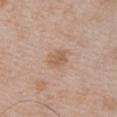| field | value |
|---|---|
| notes | no biopsy performed (imaged during a skin exam) |
| acquisition | 15 mm crop, total-body photography |
| subject | male, aged around 50 |
| tile lighting | white-light |
| site | the chest |
| lesion diameter | ~2.5 mm (longest diameter) |
| TBP lesion metrics | an average lesion color of about L≈58 a*≈18 b*≈31 (CIELAB), roughly 8 lightness units darker than nearby skin, and a lesion-to-skin contrast of about 6 (normalized; higher = more distinct); a border-irregularity index near 2/10 and a peripheral color-asymmetry measure near 1; a nevus-likeness score of about 10/100 and a lesion-detection confidence of about 100/100 |